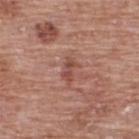The lesion was tiled from a total-body skin photograph and was not biopsied.
The tile uses white-light illumination.
The lesion is located on the upper back.
A female subject, aged 58–62.
About 3 mm across.
A roughly 15 mm field-of-view crop from a total-body skin photograph.